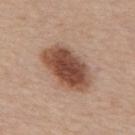{
  "biopsy_status": "not biopsied; imaged during a skin examination"
}